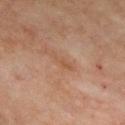The lesion's longest dimension is about 3.5 mm. The tile uses cross-polarized illumination. From the chest. A male subject aged 53 to 57. A 15 mm close-up extracted from a 3D total-body photography capture.